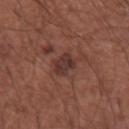Part of a total-body skin-imaging series; this lesion was reviewed on a skin check and was not flagged for biopsy. A 15 mm crop from a total-body photograph taken for skin-cancer surveillance. Located on the left upper arm. The subject is a male aged around 45.